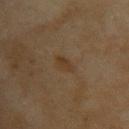follow-up — imaged on a skin check; not biopsied
size — about 3 mm
patient — female, roughly 60 years of age
site — the upper back
image source — ~15 mm tile from a whole-body skin photo
illumination — cross-polarized illumination
automated metrics — an average lesion color of about L≈33 a*≈13 b*≈28 (CIELAB), a lesion–skin lightness drop of about 5, and a lesion-to-skin contrast of about 6 (normalized; higher = more distinct); a border-irregularity index near 3/10, a within-lesion color-variation index near 1.5/10, and radial color variation of about 0.5; a classifier nevus-likeness of about 10/100 and a detector confidence of about 100 out of 100 that the crop contains a lesion